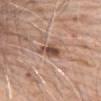The lesion was tiled from a total-body skin photograph and was not biopsied.
The lesion is located on the abdomen.
Captured under white-light illumination.
A region of skin cropped from a whole-body photographic capture, roughly 15 mm wide.
Measured at roughly 4 mm in maximum diameter.
The lesion-visualizer software estimated a mean CIELAB color near L≈50 a*≈20 b*≈27 and about 14 CIELAB-L* units darker than the surrounding skin.
The patient is a male roughly 80 years of age.